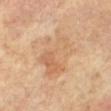Case summary:
• biopsy status · total-body-photography surveillance lesion; no biopsy
• subject · female, aged 63 to 67
• location · the right leg
• diameter · about 7 mm
• imaging modality · ~15 mm crop, total-body skin-cancer survey